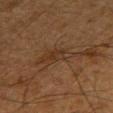{
  "biopsy_status": "not biopsied; imaged during a skin examination",
  "site": "chest",
  "patient": {
    "sex": "male",
    "age_approx": 65
  },
  "lesion_size": {
    "long_diameter_mm_approx": 5.0
  },
  "automated_metrics": {
    "area_mm2_approx": 8.0,
    "eccentricity": 0.85,
    "vs_skin_darker_L": 5.0,
    "vs_skin_contrast_norm": 5.0,
    "border_irregularity_0_10": 4.5,
    "color_variation_0_10": 3.0,
    "peripheral_color_asymmetry": 1.0
  },
  "lighting": "cross-polarized",
  "image": {
    "source": "total-body photography crop",
    "field_of_view_mm": 15
  }
}